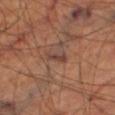Located on the leg.
Captured under cross-polarized illumination.
The lesion's longest dimension is about 2.5 mm.
The patient is a male aged approximately 70.
A 15 mm crop from a total-body photograph taken for skin-cancer surveillance.